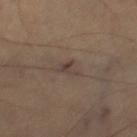notes: total-body-photography surveillance lesion; no biopsy
image: ~15 mm crop, total-body skin-cancer survey
patient: male, approximately 65 years of age
anatomic site: the right thigh
size: about 2.5 mm
tile lighting: cross-polarized illumination